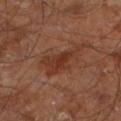biopsy status: imaged on a skin check; not biopsied | anatomic site: the right leg | lesion size: about 5 mm | illumination: cross-polarized illumination | subject: male, roughly 60 years of age | acquisition: ~15 mm tile from a whole-body skin photo | image-analysis metrics: a mean CIELAB color near L≈33 a*≈22 b*≈28, about 7 CIELAB-L* units darker than the surrounding skin, and a lesion-to-skin contrast of about 7 (normalized; higher = more distinct); a border-irregularity rating of about 6.5/10, a color-variation rating of about 3/10, and peripheral color asymmetry of about 1; an automated nevus-likeness rating near 0 out of 100 and a lesion-detection confidence of about 100/100.